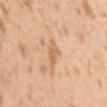Clinical impression:
No biopsy was performed on this lesion — it was imaged during a full skin examination and was not determined to be concerning.
Image and clinical context:
The patient is a male roughly 25 years of age. The tile uses white-light illumination. The lesion is located on the left upper arm. About 2.5 mm across. The total-body-photography lesion software estimated an area of roughly 4 mm² and an eccentricity of roughly 0.8. It also reported an average lesion color of about L≈66 a*≈22 b*≈37 (CIELAB) and about 8 CIELAB-L* units darker than the surrounding skin. The analysis additionally found a classifier nevus-likeness of about 0/100 and a detector confidence of about 100 out of 100 that the crop contains a lesion. A roughly 15 mm field-of-view crop from a total-body skin photograph.